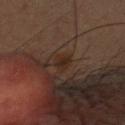Part of a total-body skin-imaging series; this lesion was reviewed on a skin check and was not flagged for biopsy. About 2.5 mm across. A male subject about 60 years old. A 15 mm close-up tile from a total-body photography series done for melanoma screening. Located on the right upper arm.